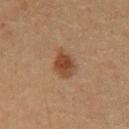Clinical impression:
Part of a total-body skin-imaging series; this lesion was reviewed on a skin check and was not flagged for biopsy.
Clinical summary:
Located on the front of the torso. Cropped from a total-body skin-imaging series; the visible field is about 15 mm. The lesion's longest dimension is about 3.5 mm. The subject is a male approximately 65 years of age.